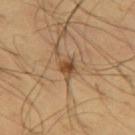Q: How was this image acquired?
A: ~15 mm tile from a whole-body skin photo
Q: Illumination type?
A: cross-polarized
Q: What are the patient's age and sex?
A: male, aged around 65
Q: Lesion location?
A: the leg
Q: What did automated image analysis measure?
A: a footprint of about 4 mm² and a shape-asymmetry score of about 0.25 (0 = symmetric); a mean CIELAB color near L≈48 a*≈19 b*≈34, roughly 12 lightness units darker than nearby skin, and a lesion-to-skin contrast of about 8.5 (normalized; higher = more distinct); border irregularity of about 2.5 on a 0–10 scale, a color-variation rating of about 4/10, and radial color variation of about 1; a classifier nevus-likeness of about 90/100 and a detector confidence of about 100 out of 100 that the crop contains a lesion
Q: How large is the lesion?
A: about 2.5 mm